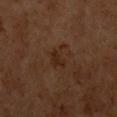| key | value |
|---|---|
| follow-up | no biopsy performed (imaged during a skin exam) |